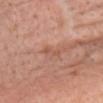| field | value |
|---|---|
| workup | imaged on a skin check; not biopsied |
| TBP lesion metrics | an eccentricity of roughly 0.95 and a shape-asymmetry score of about 0.55 (0 = symmetric); an average lesion color of about L≈55 a*≈23 b*≈31 (CIELAB), a lesion–skin lightness drop of about 7, and a lesion-to-skin contrast of about 5.5 (normalized; higher = more distinct); border irregularity of about 6.5 on a 0–10 scale and internal color variation of about 0 on a 0–10 scale; a lesion-detection confidence of about 100/100 |
| image | ~15 mm tile from a whole-body skin photo |
| location | the front of the torso |
| patient | male, aged 73–77 |
| lighting | white-light illumination |